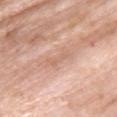Q: Was a biopsy performed?
A: no biopsy performed (imaged during a skin exam)
Q: How was this image acquired?
A: 15 mm crop, total-body photography
Q: Lesion location?
A: the back
Q: What lighting was used for the tile?
A: white-light illumination
Q: Lesion size?
A: about 3.5 mm
Q: What are the patient's age and sex?
A: female, in their 70s